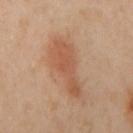The lesion was photographed on a routine skin check and not biopsied; there is no pathology result. Located on the left arm. A female patient, aged approximately 40. A 15 mm close-up tile from a total-body photography series done for melanoma screening.